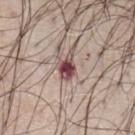workup: imaged on a skin check; not biopsied | lighting: white-light | acquisition: 15 mm crop, total-body photography | site: the chest | patient: male, approximately 70 years of age | lesion diameter: ≈3.5 mm.